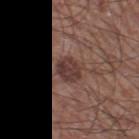• workup: total-body-photography surveillance lesion; no biopsy
• location: the left thigh
• illumination: white-light
• acquisition: ~15 mm tile from a whole-body skin photo
• patient: male, aged 58–62
• lesion diameter: ≈3.5 mm